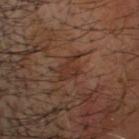Assessment:
No biopsy was performed on this lesion — it was imaged during a full skin examination and was not determined to be concerning.
Background:
Captured under cross-polarized illumination. An algorithmic analysis of the crop reported a lesion area of about 4.5 mm², an outline eccentricity of about 0.7 (0 = round, 1 = elongated), and a shape-asymmetry score of about 0.45 (0 = symmetric). And it measured a lesion–skin lightness drop of about 6 and a normalized lesion–skin contrast near 6. The analysis additionally found a detector confidence of about 75 out of 100 that the crop contains a lesion. A 15 mm close-up tile from a total-body photography series done for melanoma screening. The recorded lesion diameter is about 2.5 mm. On the head or neck. A male subject in their mid- to late 60s.